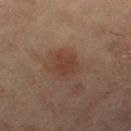* follow-up · no biopsy performed (imaged during a skin exam)
* acquisition · ~15 mm crop, total-body skin-cancer survey
* automated metrics · a footprint of about 9.5 mm², an outline eccentricity of about 0.45 (0 = round, 1 = elongated), and a shape-asymmetry score of about 0.25 (0 = symmetric); a mean CIELAB color near L≈34 a*≈17 b*≈23, about 6 CIELAB-L* units darker than the surrounding skin, and a normalized lesion–skin contrast near 6; a nevus-likeness score of about 50/100 and lesion-presence confidence of about 100/100
* body site · the right thigh
* lighting · cross-polarized illumination
* subject · female, aged around 55
* lesion diameter · about 4 mm